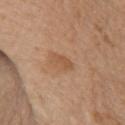Notes:
• follow-up — imaged on a skin check; not biopsied
• patient — male, in their mid-70s
• site — the chest
• diameter — about 3 mm
• illumination — white-light illumination
• automated lesion analysis — an area of roughly 4 mm², an eccentricity of roughly 0.8, and a symmetry-axis asymmetry near 0.2; a lesion–skin lightness drop of about 7 and a lesion-to-skin contrast of about 5.5 (normalized; higher = more distinct); a within-lesion color-variation index near 2/10 and radial color variation of about 0.5; a detector confidence of about 100 out of 100 that the crop contains a lesion
• acquisition — total-body-photography crop, ~15 mm field of view